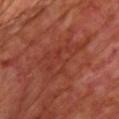Captured during whole-body skin photography for melanoma surveillance; the lesion was not biopsied. The tile uses cross-polarized illumination. A male patient, in their 70s. The lesion is located on the front of the torso. This image is a 15 mm lesion crop taken from a total-body photograph. About 8 mm across.